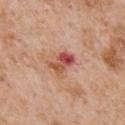Case summary:
– follow-up · total-body-photography surveillance lesion; no biopsy
– size · about 3.5 mm
– subject · male, aged 63 to 67
– tile lighting · white-light illumination
– acquisition · ~15 mm crop, total-body skin-cancer survey
– anatomic site · the chest